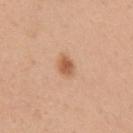No biopsy was performed on this lesion — it was imaged during a full skin examination and was not determined to be concerning. The lesion is on the right upper arm. A female patient, about 30 years old. This image is a 15 mm lesion crop taken from a total-body photograph. This is a white-light tile.